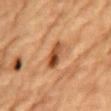notes = imaged on a skin check; not biopsied | image source = 15 mm crop, total-body photography | lighting = cross-polarized illumination | patient = male, aged around 85 or older | location = the chest.